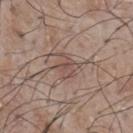| field | value |
|---|---|
| follow-up | no biopsy performed (imaged during a skin exam) |
| lesion size | ≈3 mm |
| subject | male, in their mid-70s |
| lighting | white-light illumination |
| body site | the chest |
| acquisition | ~15 mm tile from a whole-body skin photo |